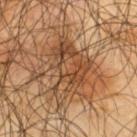follow-up: no biopsy performed (imaged during a skin exam) | size: ≈8 mm | illumination: cross-polarized | imaging modality: ~15 mm crop, total-body skin-cancer survey | subject: male, in their mid- to late 60s | automated metrics: a mean CIELAB color near L≈44 a*≈19 b*≈33, about 11 CIELAB-L* units darker than the surrounding skin, and a normalized border contrast of about 8.5; a border-irregularity index near 6/10, a color-variation rating of about 8.5/10, and radial color variation of about 3.5 | body site: the upper back.